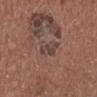Assessment: The lesion was photographed on a routine skin check and not biopsied; there is no pathology result. Context: The lesion is on the left lower leg. A close-up tile cropped from a whole-body skin photograph, about 15 mm across. The lesion's longest dimension is about 3.5 mm. The tile uses white-light illumination. A female patient roughly 50 years of age.